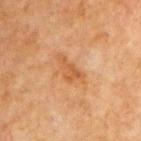Imaged during a routine full-body skin examination; the lesion was not biopsied and no histopathology is available. A male subject aged around 65. On the chest. Cropped from a total-body skin-imaging series; the visible field is about 15 mm.